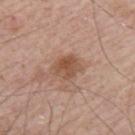follow-up — imaged on a skin check; not biopsied | lighting — white-light | anatomic site — the left upper arm | diameter — ≈3.5 mm | subject — male, in their mid-60s | image — total-body-photography crop, ~15 mm field of view.